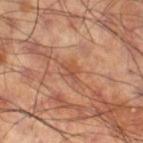No biopsy was performed on this lesion — it was imaged during a full skin examination and was not determined to be concerning. Located on the right thigh. A 15 mm close-up tile from a total-body photography series done for melanoma screening. Longest diameter approximately 2.5 mm. Captured under cross-polarized illumination. The subject is a male approximately 60 years of age.